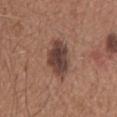| key | value |
|---|---|
| follow-up | catalogued during a skin exam; not biopsied |
| lesion diameter | ~6 mm (longest diameter) |
| imaging modality | ~15 mm crop, total-body skin-cancer survey |
| anatomic site | the mid back |
| patient | male, aged approximately 65 |
| illumination | white-light illumination |